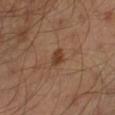The lesion was photographed on a routine skin check and not biopsied; there is no pathology result. The recorded lesion diameter is about 2 mm. A region of skin cropped from a whole-body photographic capture, roughly 15 mm wide. Imaged with cross-polarized lighting. A male patient aged around 45. An algorithmic analysis of the crop reported an area of roughly 3 mm², a shape eccentricity near 0.5, and a symmetry-axis asymmetry near 0.2. The software also gave a nevus-likeness score of about 85/100. On the leg.